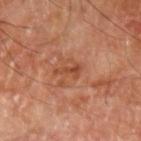Q: Was a biopsy performed?
A: no biopsy performed (imaged during a skin exam)
Q: Who is the patient?
A: male, aged 68–72
Q: What is the anatomic site?
A: the left upper arm
Q: What kind of image is this?
A: 15 mm crop, total-body photography